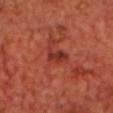Q: Lesion location?
A: the chest
Q: Illumination type?
A: cross-polarized illumination
Q: Automated lesion metrics?
A: a nevus-likeness score of about 0/100 and a lesion-detection confidence of about 100/100
Q: Patient demographics?
A: male, roughly 70 years of age
Q: What is the imaging modality?
A: 15 mm crop, total-body photography
Q: What is the lesion's diameter?
A: about 3 mm